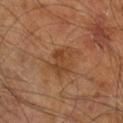Clinical impression:
No biopsy was performed on this lesion — it was imaged during a full skin examination and was not determined to be concerning.
Image and clinical context:
A roughly 15 mm field-of-view crop from a total-body skin photograph. On the right leg. The patient is a male aged 58 to 62. The lesion's longest dimension is about 4 mm. Captured under cross-polarized illumination. Automated image analysis of the tile measured an area of roughly 9 mm², an outline eccentricity of about 0.6 (0 = round, 1 = elongated), and a symmetry-axis asymmetry near 0.3. It also reported border irregularity of about 3.5 on a 0–10 scale, a within-lesion color-variation index near 3.5/10, and a peripheral color-asymmetry measure near 1. The software also gave a nevus-likeness score of about 0/100.